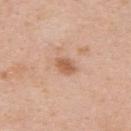Impression: Imaged during a routine full-body skin examination; the lesion was not biopsied and no histopathology is available. Background: This is a white-light tile. A female subject in their mid-30s. From the upper back. Automated tile analysis of the lesion measured a footprint of about 4 mm², a shape eccentricity near 0.7, and a shape-asymmetry score of about 0.2 (0 = symmetric). And it measured an average lesion color of about L≈59 a*≈22 b*≈34 (CIELAB), a lesion–skin lightness drop of about 11, and a normalized lesion–skin contrast near 7.5. It also reported border irregularity of about 2 on a 0–10 scale, a color-variation rating of about 1.5/10, and radial color variation of about 0.5. The analysis additionally found a nevus-likeness score of about 65/100 and a lesion-detection confidence of about 100/100. A 15 mm crop from a total-body photograph taken for skin-cancer surveillance. Longest diameter approximately 2.5 mm.